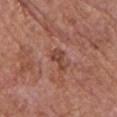workup: imaged on a skin check; not biopsied | image source: 15 mm crop, total-body photography | subject: female, aged 63–67 | diameter: ~3 mm (longest diameter) | site: the chest | illumination: white-light illumination.